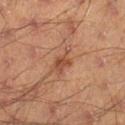Recorded during total-body skin imaging; not selected for excision or biopsy. A male patient, approximately 45 years of age. A close-up tile cropped from a whole-body skin photograph, about 15 mm across. Captured under cross-polarized illumination. The lesion is on the right lower leg. An algorithmic analysis of the crop reported an automated nevus-likeness rating near 0 out of 100 and a detector confidence of about 100 out of 100 that the crop contains a lesion. The recorded lesion diameter is about 4 mm.